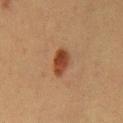Clinical impression: Imaged during a routine full-body skin examination; the lesion was not biopsied and no histopathology is available. Context: This is a cross-polarized tile. The total-body-photography lesion software estimated an area of roughly 6 mm², an eccentricity of roughly 0.8, and a shape-asymmetry score of about 0.2 (0 = symmetric). The software also gave a border-irregularity index near 2/10 and radial color variation of about 1.5. It also reported an automated nevus-likeness rating near 100 out of 100 and lesion-presence confidence of about 100/100. The patient is a female aged approximately 40. About 3.5 mm across. A roughly 15 mm field-of-view crop from a total-body skin photograph. From the front of the torso.